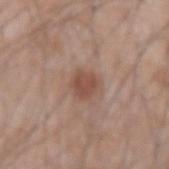notes = no biopsy performed (imaged during a skin exam)
imaging modality = 15 mm crop, total-body photography
subject = male, aged 43 to 47
tile lighting = white-light illumination
body site = the left forearm
automated metrics = a shape eccentricity near 0.35 and two-axis asymmetry of about 0.15; a nevus-likeness score of about 80/100
size = about 2.5 mm